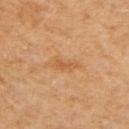No biopsy was performed on this lesion — it was imaged during a full skin examination and was not determined to be concerning. The lesion is located on the right upper arm. Approximately 3 mm at its widest. The patient is a female aged 48 to 52. A lesion tile, about 15 mm wide, cut from a 3D total-body photograph.